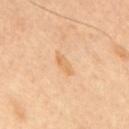Clinical impression: This lesion was catalogued during total-body skin photography and was not selected for biopsy. Clinical summary: Automated tile analysis of the lesion measured a mean CIELAB color near L≈67 a*≈20 b*≈41. Imaged with cross-polarized lighting. A male patient, about 60 years old. Approximately 3 mm at its widest. Cropped from a whole-body photographic skin survey; the tile spans about 15 mm. The lesion is located on the back.